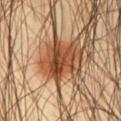<lesion>
  <biopsy_status>not biopsied; imaged during a skin examination</biopsy_status>
  <lesion_size>
    <long_diameter_mm_approx>5.0</long_diameter_mm_approx>
  </lesion_size>
  <image>
    <source>total-body photography crop</source>
    <field_of_view_mm>15</field_of_view_mm>
  </image>
  <patient>
    <sex>male</sex>
    <age_approx>45</age_approx>
  </patient>
  <site>right thigh</site>
</lesion>